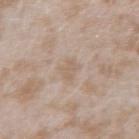Recorded during total-body skin imaging; not selected for excision or biopsy.
Approximately 2.5 mm at its widest.
On the left forearm.
A roughly 15 mm field-of-view crop from a total-body skin photograph.
An algorithmic analysis of the crop reported a detector confidence of about 100 out of 100 that the crop contains a lesion.
A female patient roughly 25 years of age.